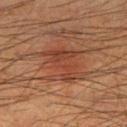notes: imaged on a skin check; not biopsied | site: the right thigh | acquisition: 15 mm crop, total-body photography | subject: male, aged around 35 | lesion diameter: ~5.5 mm (longest diameter) | illumination: cross-polarized illumination.